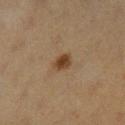Captured during whole-body skin photography for melanoma surveillance; the lesion was not biopsied. A 15 mm close-up tile from a total-body photography series done for melanoma screening. This is a cross-polarized tile. The lesion is on the left lower leg. A female patient, in their 40s.